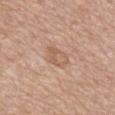Captured during whole-body skin photography for melanoma surveillance; the lesion was not biopsied.
Measured at roughly 3.5 mm in maximum diameter.
On the mid back.
A 15 mm close-up extracted from a 3D total-body photography capture.
Imaged with white-light lighting.
The subject is a male about 80 years old.